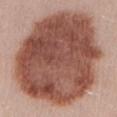Impression: Part of a total-body skin-imaging series; this lesion was reviewed on a skin check and was not flagged for biopsy. Clinical summary: The tile uses white-light illumination. A 15 mm close-up tile from a total-body photography series done for melanoma screening. The patient is a female aged 28–32. About 12.5 mm across. The lesion is located on the abdomen. Automated image analysis of the tile measured a border-irregularity rating of about 1.5/10 and radial color variation of about 2.5.